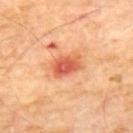Imaged during a routine full-body skin examination; the lesion was not biopsied and no histopathology is available. The recorded lesion diameter is about 3.5 mm. The total-body-photography lesion software estimated two-axis asymmetry of about 0.15. The analysis additionally found an average lesion color of about L≈59 a*≈34 b*≈39 (CIELAB), about 13 CIELAB-L* units darker than the surrounding skin, and a lesion-to-skin contrast of about 8.5 (normalized; higher = more distinct). A male subject, roughly 70 years of age. From the mid back. A 15 mm close-up tile from a total-body photography series done for melanoma screening.